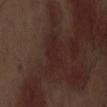Imaged during a routine full-body skin examination; the lesion was not biopsied and no histopathology is available.
The lesion is located on the abdomen.
Automated tile analysis of the lesion measured an automated nevus-likeness rating near 0 out of 100 and a lesion-detection confidence of about 90/100.
Approximately 4.5 mm at its widest.
A 15 mm crop from a total-body photograph taken for skin-cancer surveillance.
A male patient aged 68–72.
Imaged with white-light lighting.